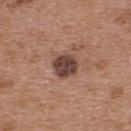biopsy status = no biopsy performed (imaged during a skin exam); subject = female, aged around 40; image source = total-body-photography crop, ~15 mm field of view; lesion diameter = ≈3 mm; body site = the upper back.